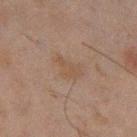No biopsy was performed on this lesion — it was imaged during a full skin examination and was not determined to be concerning. A male subject aged 43 to 47. This is a cross-polarized tile. About 4 mm across. The lesion is on the left thigh. A roughly 15 mm field-of-view crop from a total-body skin photograph.